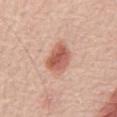notes: catalogued during a skin exam; not biopsied | patient: male, in their 70s | imaging modality: ~15 mm crop, total-body skin-cancer survey | automated lesion analysis: a lesion color around L≈58 a*≈27 b*≈30 in CIELAB, roughly 13 lightness units darker than nearby skin, and a normalized border contrast of about 8.5; a classifier nevus-likeness of about 100/100 | body site: the mid back.